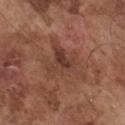Findings:
• notes · catalogued during a skin exam; not biopsied
• patient · male, roughly 75 years of age
• automated metrics · a lesion area of about 13 mm² and a shape-asymmetry score of about 0.6 (0 = symmetric)
• anatomic site · the chest
• imaging modality · ~15 mm tile from a whole-body skin photo
• lighting · white-light illumination